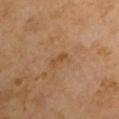Impression: Imaged during a routine full-body skin examination; the lesion was not biopsied and no histopathology is available. Image and clinical context: The patient is a male in their mid-60s. About 2.5 mm across. A 15 mm crop from a total-body photograph taken for skin-cancer surveillance. Captured under cross-polarized illumination. From the left arm.